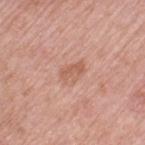<record>
<biopsy_status>not biopsied; imaged during a skin examination</biopsy_status>
<patient>
  <sex>female</sex>
  <age_approx>70</age_approx>
</patient>
<lesion_size>
  <long_diameter_mm_approx>3.0</long_diameter_mm_approx>
</lesion_size>
<image>
  <source>total-body photography crop</source>
  <field_of_view_mm>15</field_of_view_mm>
</image>
<site>left thigh</site>
</record>